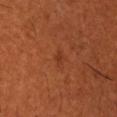Impression: The lesion was photographed on a routine skin check and not biopsied; there is no pathology result. Background: The tile uses cross-polarized illumination. On the head or neck. The lesion's longest dimension is about 1 mm. The total-body-photography lesion software estimated an area of roughly 1 mm², an eccentricity of roughly 0.6, and a shape-asymmetry score of about 0.4 (0 = symmetric). The software also gave an average lesion color of about L≈32 a*≈26 b*≈32 (CIELAB), about 6 CIELAB-L* units darker than the surrounding skin, and a normalized lesion–skin contrast near 5.5. And it measured a within-lesion color-variation index near 0/10 and a peripheral color-asymmetry measure near 0. And it measured an automated nevus-likeness rating near 0 out of 100 and a detector confidence of about 100 out of 100 that the crop contains a lesion. A male patient, aged 58 to 62. A lesion tile, about 15 mm wide, cut from a 3D total-body photograph.